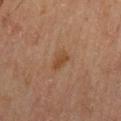biopsy status = total-body-photography surveillance lesion; no biopsy
acquisition = 15 mm crop, total-body photography
subject = male, aged 68 to 72
body site = the mid back
diameter = ~2.5 mm (longest diameter)
lighting = cross-polarized